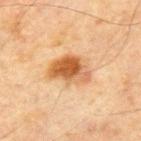{"biopsy_status": "not biopsied; imaged during a skin examination", "lighting": "cross-polarized", "patient": {"sex": "male", "age_approx": 70}, "site": "chest", "lesion_size": {"long_diameter_mm_approx": 5.0}, "automated_metrics": {"border_irregularity_0_10": 2.5, "color_variation_0_10": 8.0, "lesion_detection_confidence_0_100": 100}, "image": {"source": "total-body photography crop", "field_of_view_mm": 15}}